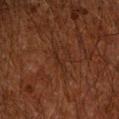Case summary:
- automated metrics: an area of roughly 2 mm², an eccentricity of roughly 0.95, and a symmetry-axis asymmetry near 0.7; about 3 CIELAB-L* units darker than the surrounding skin; a classifier nevus-likeness of about 0/100 and a lesion-detection confidence of about 60/100
- illumination: cross-polarized illumination
- image: ~15 mm tile from a whole-body skin photo
- subject: male, about 60 years old
- lesion size: ~3.5 mm (longest diameter)
- location: the arm